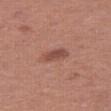Captured during whole-body skin photography for melanoma surveillance; the lesion was not biopsied.
Cropped from a whole-body photographic skin survey; the tile spans about 15 mm.
Automated image analysis of the tile measured a footprint of about 4.5 mm² and an eccentricity of roughly 0.85. The analysis additionally found a mean CIELAB color near L≈48 a*≈24 b*≈26, about 10 CIELAB-L* units darker than the surrounding skin, and a lesion-to-skin contrast of about 7 (normalized; higher = more distinct).
Captured under white-light illumination.
Located on the right thigh.
The patient is a female roughly 40 years of age.